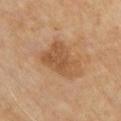Captured during whole-body skin photography for melanoma surveillance; the lesion was not biopsied.